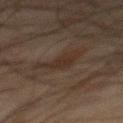Q: Was a biopsy performed?
A: no biopsy performed (imaged during a skin exam)
Q: How was this image acquired?
A: total-body-photography crop, ~15 mm field of view
Q: What are the patient's age and sex?
A: male, aged 43–47
Q: Lesion location?
A: the mid back